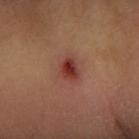Approximately 3 mm at its widest.
The lesion is on the arm.
The subject is a female about 55 years old.
This is a cross-polarized tile.
A lesion tile, about 15 mm wide, cut from a 3D total-body photograph.